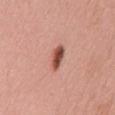workup — total-body-photography surveillance lesion; no biopsy
body site — the mid back
patient — female, roughly 70 years of age
image — ~15 mm crop, total-body skin-cancer survey
lighting — white-light
size — ~3 mm (longest diameter)